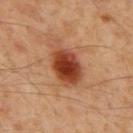Q: Was this lesion biopsied?
A: imaged on a skin check; not biopsied
Q: Lesion location?
A: the mid back
Q: What are the patient's age and sex?
A: male, aged 58–62
Q: How was the tile lit?
A: cross-polarized illumination
Q: What kind of image is this?
A: total-body-photography crop, ~15 mm field of view
Q: Lesion size?
A: ~5.5 mm (longest diameter)